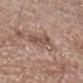Q: How was this image acquired?
A: ~15 mm tile from a whole-body skin photo
Q: What are the patient's age and sex?
A: male, aged 78–82
Q: Where on the body is the lesion?
A: the front of the torso
Q: Lesion size?
A: about 4 mm
Q: How was the tile lit?
A: white-light illumination